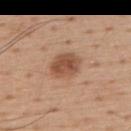| field | value |
|---|---|
| workup | no biopsy performed (imaged during a skin exam) |
| lesion size | about 3.5 mm |
| illumination | white-light |
| imaging modality | 15 mm crop, total-body photography |
| site | the upper back |
| TBP lesion metrics | a mean CIELAB color near L≈51 a*≈22 b*≈31 and a lesion-to-skin contrast of about 8.5 (normalized; higher = more distinct); an automated nevus-likeness rating near 95 out of 100 and lesion-presence confidence of about 100/100 |
| subject | male, approximately 55 years of age |